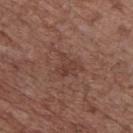notes — total-body-photography surveillance lesion; no biopsy | body site — the chest | acquisition — 15 mm crop, total-body photography | lighting — white-light | lesion diameter — ≈2.5 mm | TBP lesion metrics — an area of roughly 4.5 mm², an outline eccentricity of about 0.35 (0 = round, 1 = elongated), and a shape-asymmetry score of about 0.55 (0 = symmetric); internal color variation of about 2.5 on a 0–10 scale and peripheral color asymmetry of about 1 | patient — male, about 70 years old.